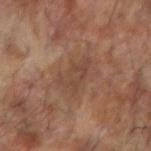Longest diameter approximately 4 mm. The tile uses cross-polarized illumination. A region of skin cropped from a whole-body photographic capture, roughly 15 mm wide. Located on the right forearm. The patient is a male roughly 65 years of age. Automated tile analysis of the lesion measured border irregularity of about 5 on a 0–10 scale, a color-variation rating of about 2.5/10, and peripheral color asymmetry of about 0.5. And it measured a nevus-likeness score of about 0/100 and a detector confidence of about 95 out of 100 that the crop contains a lesion.